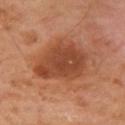Q: Was a biopsy performed?
A: imaged on a skin check; not biopsied
Q: Lesion location?
A: the right upper arm
Q: What are the patient's age and sex?
A: female, approximately 55 years of age
Q: What is the imaging modality?
A: ~15 mm tile from a whole-body skin photo